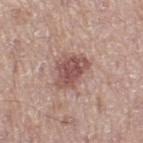| feature | finding |
|---|---|
| follow-up | no biopsy performed (imaged during a skin exam) |
| site | the left thigh |
| imaging modality | ~15 mm crop, total-body skin-cancer survey |
| diameter | ≈4.5 mm |
| TBP lesion metrics | a shape-asymmetry score of about 0.3 (0 = symmetric); an automated nevus-likeness rating near 65 out of 100 and a detector confidence of about 100 out of 100 that the crop contains a lesion |
| illumination | white-light |
| subject | male, aged approximately 65 |